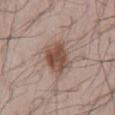No biopsy was performed on this lesion — it was imaged during a full skin examination and was not determined to be concerning. On the lower back. A 15 mm close-up extracted from a 3D total-body photography capture. Measured at roughly 4 mm in maximum diameter. Imaged with white-light lighting. A male subject roughly 40 years of age. An algorithmic analysis of the crop reported an eccentricity of roughly 0.45 and a symmetry-axis asymmetry near 0.15. The software also gave a classifier nevus-likeness of about 90/100 and a lesion-detection confidence of about 100/100.